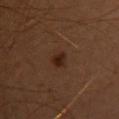imaging modality: ~15 mm tile from a whole-body skin photo | illumination: cross-polarized | diameter: about 2.5 mm | automated lesion analysis: an area of roughly 3.5 mm², an outline eccentricity of about 0.8 (0 = round, 1 = elongated), and a shape-asymmetry score of about 0.25 (0 = symmetric); a mean CIELAB color near L≈20 a*≈17 b*≈22 and about 7 CIELAB-L* units darker than the surrounding skin; a border-irregularity rating of about 2.5/10 and a color-variation rating of about 1.5/10 | site: the chest | patient: female, approximately 50 years of age.